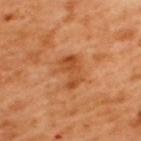biopsy status: imaged on a skin check; not biopsied
site: the upper back
acquisition: total-body-photography crop, ~15 mm field of view
lighting: cross-polarized
lesion size: ≈3.5 mm
patient: male, aged 48 to 52